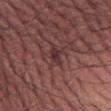{"biopsy_status": "not biopsied; imaged during a skin examination", "patient": {"sex": "male", "age_approx": 45}, "image": {"source": "total-body photography crop", "field_of_view_mm": 15}, "site": "left forearm"}